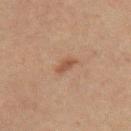<lesion>
  <biopsy_status>not biopsied; imaged during a skin examination</biopsy_status>
  <lesion_size>
    <long_diameter_mm_approx>3.0</long_diameter_mm_approx>
  </lesion_size>
  <site>arm</site>
  <lighting>cross-polarized</lighting>
  <image>
    <source>total-body photography crop</source>
    <field_of_view_mm>15</field_of_view_mm>
  </image>
  <patient>
    <sex>male</sex>
    <age_approx>30</age_approx>
  </patient>
</lesion>